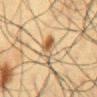A 15 mm close-up tile from a total-body photography series done for melanoma screening. This is a cross-polarized tile. A male patient aged 58 to 62. The total-body-photography lesion software estimated a lesion color around L≈45 a*≈13 b*≈29 in CIELAB, a lesion–skin lightness drop of about 10, and a lesion-to-skin contrast of about 8 (normalized; higher = more distinct). Measured at roughly 4 mm in maximum diameter. From the chest.